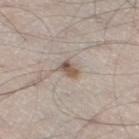Clinical impression: Part of a total-body skin-imaging series; this lesion was reviewed on a skin check and was not flagged for biopsy. Acquisition and patient details: The recorded lesion diameter is about 2.5 mm. The subject is a male aged around 40. A region of skin cropped from a whole-body photographic capture, roughly 15 mm wide. Captured under white-light illumination. On the right thigh.